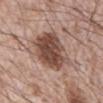The lesion was photographed on a routine skin check and not biopsied; there is no pathology result. The lesion is on the abdomen. The tile uses white-light illumination. About 5.5 mm across. A male patient aged 68 to 72. A 15 mm close-up tile from a total-body photography series done for melanoma screening.